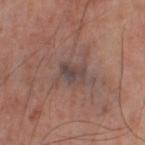  biopsy_status: not biopsied; imaged during a skin examination
  lesion_size:
    long_diameter_mm_approx: 2.5
  image:
    source: total-body photography crop
    field_of_view_mm: 15
  automated_metrics:
    vs_skin_darker_L: 8.0
    border_irregularity_0_10: 4.0
    color_variation_0_10: 3.0
    peripheral_color_asymmetry: 1.0
  lighting: cross-polarized
  site: right thigh